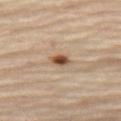workup: total-body-photography surveillance lesion; no biopsy
tile lighting: cross-polarized illumination
imaging modality: ~15 mm tile from a whole-body skin photo
lesion size: ~2.5 mm (longest diameter)
TBP lesion metrics: a lesion area of about 3.5 mm² and an outline eccentricity of about 0.75 (0 = round, 1 = elongated); a lesion color around L≈52 a*≈19 b*≈32 in CIELAB, a lesion–skin lightness drop of about 15, and a lesion-to-skin contrast of about 10.5 (normalized; higher = more distinct); an automated nevus-likeness rating near 95 out of 100 and a detector confidence of about 100 out of 100 that the crop contains a lesion
subject: female, aged approximately 65
location: the left thigh